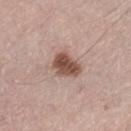The lesion was tiled from a total-body skin photograph and was not biopsied.
Automated image analysis of the tile measured an average lesion color of about L≈50 a*≈20 b*≈25 (CIELAB), about 15 CIELAB-L* units darker than the surrounding skin, and a normalized border contrast of about 10.5. The analysis additionally found a border-irregularity rating of about 2.5/10 and radial color variation of about 1.5. The analysis additionally found a classifier nevus-likeness of about 95/100.
The recorded lesion diameter is about 3.5 mm.
Imaged with white-light lighting.
The lesion is located on the left thigh.
A male subject aged around 75.
A roughly 15 mm field-of-view crop from a total-body skin photograph.